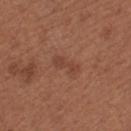Q: Was a biopsy performed?
A: imaged on a skin check; not biopsied
Q: Lesion location?
A: the left thigh
Q: What are the patient's age and sex?
A: female, approximately 55 years of age
Q: What is the imaging modality?
A: 15 mm crop, total-body photography